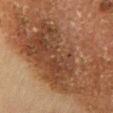workup — imaged on a skin check; not biopsied | image source — ~15 mm crop, total-body skin-cancer survey | location — the chest | tile lighting — cross-polarized illumination | patient — female, aged approximately 65 | lesion diameter — about 12.5 mm.